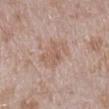Captured during whole-body skin photography for melanoma surveillance; the lesion was not biopsied. Approximately 4.5 mm at its widest. A male subject, aged approximately 45. A close-up tile cropped from a whole-body skin photograph, about 15 mm across. Located on the leg. Captured under white-light illumination. Automated tile analysis of the lesion measured a mean CIELAB color near L≈59 a*≈17 b*≈26 and about 7 CIELAB-L* units darker than the surrounding skin. It also reported a border-irregularity rating of about 4/10.